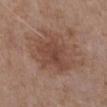The lesion was photographed on a routine skin check and not biopsied; there is no pathology result. This is a white-light tile. The recorded lesion diameter is about 6 mm. Automated tile analysis of the lesion measured a border-irregularity rating of about 3.5/10 and a peripheral color-asymmetry measure near 1. The software also gave a classifier nevus-likeness of about 15/100 and lesion-presence confidence of about 100/100. On the left upper arm. A male subject, aged approximately 50. Cropped from a total-body skin-imaging series; the visible field is about 15 mm.